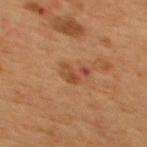The lesion was photographed on a routine skin check and not biopsied; there is no pathology result. The subject is a male approximately 55 years of age. Cropped from a whole-body photographic skin survey; the tile spans about 15 mm. About 3 mm across. An algorithmic analysis of the crop reported a shape eccentricity near 0.75 and two-axis asymmetry of about 0.5. The analysis additionally found an automated nevus-likeness rating near 5 out of 100. Imaged with cross-polarized lighting. Located on the mid back.